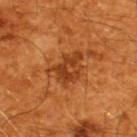Q: Is there a histopathology result?
A: catalogued during a skin exam; not biopsied
Q: Lesion size?
A: about 4.5 mm
Q: Automated lesion metrics?
A: a footprint of about 9.5 mm² and a symmetry-axis asymmetry near 0.45; a lesion color around L≈38 a*≈27 b*≈39 in CIELAB; border irregularity of about 5 on a 0–10 scale, a within-lesion color-variation index near 4/10, and peripheral color asymmetry of about 1.5
Q: Patient demographics?
A: male, in their 60s
Q: Where on the body is the lesion?
A: the upper back
Q: What kind of image is this?
A: ~15 mm crop, total-body skin-cancer survey
Q: How was the tile lit?
A: cross-polarized illumination